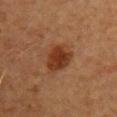This is a cross-polarized tile.
Approximately 4 mm at its widest.
The lesion is on the chest.
This image is a 15 mm lesion crop taken from a total-body photograph.
A female subject aged around 50.